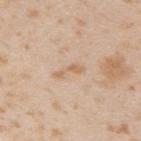Assessment: Imaged during a routine full-body skin examination; the lesion was not biopsied and no histopathology is available. Background: Cropped from a total-body skin-imaging series; the visible field is about 15 mm. The subject is a male in their mid- to late 20s. The lesion is on the right upper arm.